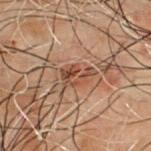  biopsy_status: not biopsied; imaged during a skin examination
  site: front of the torso
  image:
    source: total-body photography crop
    field_of_view_mm: 15
  patient:
    sex: male
    age_approx: 50